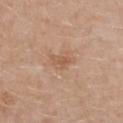{"lesion_size": {"long_diameter_mm_approx": 2.5}, "lighting": "white-light", "image": {"source": "total-body photography crop", "field_of_view_mm": 15}, "patient": {"sex": "female", "age_approx": 30}, "site": "front of the torso", "automated_metrics": {"area_mm2_approx": 3.5, "shape_asymmetry": 0.35, "cielab_L": 56, "cielab_a": 20, "cielab_b": 32, "vs_skin_darker_L": 7.0, "vs_skin_contrast_norm": 5.5, "border_irregularity_0_10": 3.0, "peripheral_color_asymmetry": 0.5}}